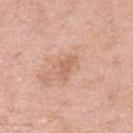Assessment: The lesion was photographed on a routine skin check and not biopsied; there is no pathology result. Clinical summary: A close-up tile cropped from a whole-body skin photograph, about 15 mm across. The lesion's longest dimension is about 3.5 mm. The lesion-visualizer software estimated an average lesion color of about L≈63 a*≈22 b*≈31 (CIELAB) and a lesion–skin lightness drop of about 7. The analysis additionally found border irregularity of about 5 on a 0–10 scale, internal color variation of about 1 on a 0–10 scale, and peripheral color asymmetry of about 0.5. The software also gave a nevus-likeness score of about 0/100 and a lesion-detection confidence of about 100/100. Captured under white-light illumination. A female subject aged around 70. From the leg.